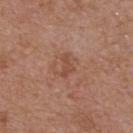Assessment:
Imaged during a routine full-body skin examination; the lesion was not biopsied and no histopathology is available.
Acquisition and patient details:
About 2.5 mm across. The lesion-visualizer software estimated a mean CIELAB color near L≈48 a*≈23 b*≈29, roughly 7 lightness units darker than nearby skin, and a lesion-to-skin contrast of about 5.5 (normalized; higher = more distinct). It also reported a border-irregularity rating of about 5/10, a within-lesion color-variation index near 0/10, and a peripheral color-asymmetry measure near 0. A close-up tile cropped from a whole-body skin photograph, about 15 mm across. The patient is a male in their mid- to late 50s. On the chest.